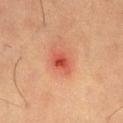Clinical impression:
The lesion was tiled from a total-body skin photograph and was not biopsied.
Image and clinical context:
The subject is a male aged 58 to 62. A 15 mm close-up extracted from a 3D total-body photography capture. The total-body-photography lesion software estimated a mean CIELAB color near L≈47 a*≈32 b*≈32, roughly 10 lightness units darker than nearby skin, and a normalized border contrast of about 7.5. The software also gave a within-lesion color-variation index near 4.5/10 and radial color variation of about 1.